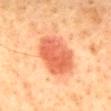Captured during whole-body skin photography for melanoma surveillance; the lesion was not biopsied.
From the mid back.
Cropped from a whole-body photographic skin survey; the tile spans about 15 mm.
A male subject about 45 years old.